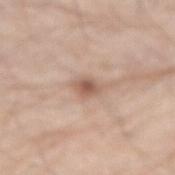Q: Is there a histopathology result?
A: imaged on a skin check; not biopsied
Q: Illumination type?
A: white-light
Q: How was this image acquired?
A: ~15 mm crop, total-body skin-cancer survey
Q: Where on the body is the lesion?
A: the left arm
Q: How large is the lesion?
A: ≈2.5 mm
Q: Patient demographics?
A: male, aged approximately 80